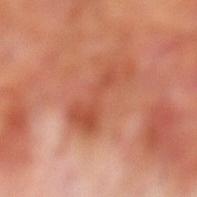biopsy status: imaged on a skin check; not biopsied
lighting: cross-polarized illumination
image-analysis metrics: a lesion-to-skin contrast of about 6 (normalized; higher = more distinct)
size: ≈6 mm
anatomic site: the left lower leg
patient: male, aged 68–72
image source: total-body-photography crop, ~15 mm field of view